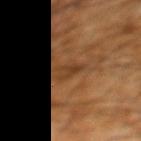This lesion was catalogued during total-body skin photography and was not selected for biopsy.
The patient is a male approximately 60 years of age.
A close-up tile cropped from a whole-body skin photograph, about 15 mm across.
The lesion is on the left lower leg.